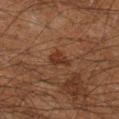{"biopsy_status": "not biopsied; imaged during a skin examination", "lighting": "cross-polarized", "image": {"source": "total-body photography crop", "field_of_view_mm": 15}, "patient": {"sex": "male", "age_approx": 60}, "site": "leg", "lesion_size": {"long_diameter_mm_approx": 3.0}}